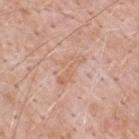The lesion was photographed on a routine skin check and not biopsied; there is no pathology result. A male patient roughly 50 years of age. A lesion tile, about 15 mm wide, cut from a 3D total-body photograph. The lesion is on the upper back.